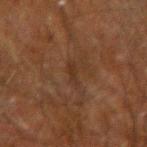notes — catalogued during a skin exam; not biopsied
anatomic site — the left forearm
size — about 2.5 mm
image — 15 mm crop, total-body photography
subject — male, aged 58 to 62
illumination — cross-polarized